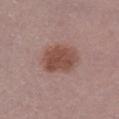Clinical impression: This lesion was catalogued during total-body skin photography and was not selected for biopsy. Acquisition and patient details: A lesion tile, about 15 mm wide, cut from a 3D total-body photograph. A male patient approximately 70 years of age. Imaged with white-light lighting. From the right lower leg.